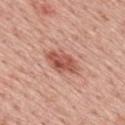Assessment: Imaged during a routine full-body skin examination; the lesion was not biopsied and no histopathology is available. Context: Automated image analysis of the tile measured a lesion area of about 8.5 mm², a shape eccentricity near 0.85, and a shape-asymmetry score of about 0.2 (0 = symmetric). It also reported a nevus-likeness score of about 90/100. The lesion is located on the upper back. The patient is a male in their 60s. This is a white-light tile. The lesion's longest dimension is about 4.5 mm. A 15 mm close-up tile from a total-body photography series done for melanoma screening.